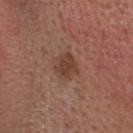The subject is a female aged 53–57. Approximately 3 mm at its widest. The tile uses white-light illumination. The lesion is on the head or neck. A 15 mm crop from a total-body photograph taken for skin-cancer surveillance.